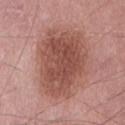{"biopsy_status": "not biopsied; imaged during a skin examination", "site": "abdomen", "lesion_size": {"long_diameter_mm_approx": 8.5}, "image": {"source": "total-body photography crop", "field_of_view_mm": 15}, "patient": {"sex": "male", "age_approx": 65}, "lighting": "white-light", "automated_metrics": {"color_variation_0_10": 4.5, "peripheral_color_asymmetry": 1.5}}